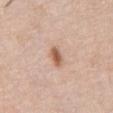The lesion was tiled from a total-body skin photograph and was not biopsied.
On the abdomen.
A 15 mm close-up tile from a total-body photography series done for melanoma screening.
A male subject, roughly 80 years of age.